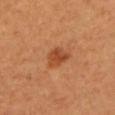This lesion was catalogued during total-body skin photography and was not selected for biopsy. The subject is a female aged around 55. The lesion is located on the chest. A region of skin cropped from a whole-body photographic capture, roughly 15 mm wide. Longest diameter approximately 3 mm.